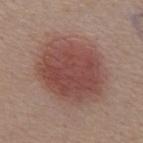| feature | finding |
|---|---|
| anatomic site | the abdomen |
| image source | ~15 mm crop, total-body skin-cancer survey |
| patient | male, aged approximately 55 |